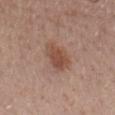Q: Was this lesion biopsied?
A: no biopsy performed (imaged during a skin exam)
Q: Where on the body is the lesion?
A: the mid back
Q: Who is the patient?
A: female, approximately 65 years of age
Q: Automated lesion metrics?
A: an area of roughly 9.5 mm², a shape eccentricity near 0.85, and two-axis asymmetry of about 0.2; a mean CIELAB color near L≈49 a*≈21 b*≈27, roughly 9 lightness units darker than nearby skin, and a normalized border contrast of about 7; lesion-presence confidence of about 100/100
Q: What kind of image is this?
A: ~15 mm crop, total-body skin-cancer survey
Q: Illumination type?
A: white-light illumination